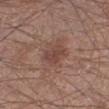notes: no biopsy performed (imaged during a skin exam); illumination: white-light; patient: male, in their 60s; image source: ~15 mm tile from a whole-body skin photo; body site: the left lower leg; size: ~3.5 mm (longest diameter).